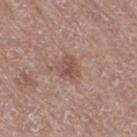workup: imaged on a skin check; not biopsied | illumination: white-light | lesion size: ≈3 mm | subject: female, aged 73 to 77 | image: ~15 mm tile from a whole-body skin photo | site: the leg.